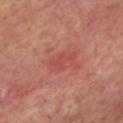workup — total-body-photography surveillance lesion; no biopsy | anatomic site — the chest | tile lighting — cross-polarized illumination | image — 15 mm crop, total-body photography | automated metrics — a lesion area of about 4 mm², an outline eccentricity of about 0.85 (0 = round, 1 = elongated), and a shape-asymmetry score of about 0.5 (0 = symmetric); a lesion–skin lightness drop of about 6 and a normalized lesion–skin contrast near 4.5; a border-irregularity index near 5/10, internal color variation of about 1 on a 0–10 scale, and radial color variation of about 0.5 | patient — approximately 55 years of age.